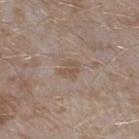Part of a total-body skin-imaging series; this lesion was reviewed on a skin check and was not flagged for biopsy. A male subject aged 43 to 47. Captured under white-light illumination. The lesion-visualizer software estimated an area of roughly 4 mm², a shape eccentricity near 0.65, and a symmetry-axis asymmetry near 0.3. It also reported a lesion color around L≈52 a*≈14 b*≈26 in CIELAB, roughly 6 lightness units darker than nearby skin, and a lesion-to-skin contrast of about 5.5 (normalized; higher = more distinct). The analysis additionally found a border-irregularity rating of about 3/10, a color-variation rating of about 2/10, and radial color variation of about 1. The analysis additionally found an automated nevus-likeness rating near 0 out of 100. This image is a 15 mm lesion crop taken from a total-body photograph. Located on the left lower leg.